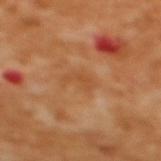Recorded during total-body skin imaging; not selected for excision or biopsy.
This image is a 15 mm lesion crop taken from a total-body photograph.
An algorithmic analysis of the crop reported a lesion color around L≈51 a*≈24 b*≈40 in CIELAB, about 6 CIELAB-L* units darker than the surrounding skin, and a normalized border contrast of about 5. The software also gave a border-irregularity index near 7/10, a within-lesion color-variation index near 0/10, and radial color variation of about 0. It also reported a classifier nevus-likeness of about 0/100 and a lesion-detection confidence of about 100/100.
From the upper back.
Imaged with cross-polarized lighting.
A female subject aged approximately 55.
Longest diameter approximately 2.5 mm.